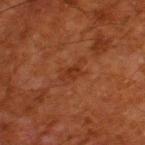Impression:
Part of a total-body skin-imaging series; this lesion was reviewed on a skin check and was not flagged for biopsy.
Context:
This image is a 15 mm lesion crop taken from a total-body photograph. This is a cross-polarized tile. The subject is a male aged 78 to 82. From the left thigh.